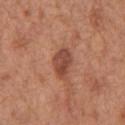site: chest
image:
  source: total-body photography crop
  field_of_view_mm: 15
patient:
  sex: male
  age_approx: 65